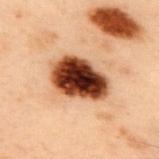This lesion was catalogued during total-body skin photography and was not selected for biopsy. The recorded lesion diameter is about 6 mm. Captured under cross-polarized illumination. Automated tile analysis of the lesion measured an average lesion color of about L≈33 a*≈23 b*≈29 (CIELAB) and a normalized lesion–skin contrast near 19.5. The software also gave a border-irregularity rating of about 2/10, internal color variation of about 10 on a 0–10 scale, and radial color variation of about 3.5. The software also gave a nevus-likeness score of about 100/100. The lesion is located on the upper back. A male patient aged 53 to 57. A close-up tile cropped from a whole-body skin photograph, about 15 mm across.